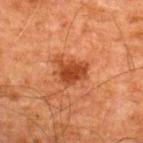workup: total-body-photography surveillance lesion; no biopsy | image source: ~15 mm tile from a whole-body skin photo | subject: male, aged around 60 | body site: the upper back.